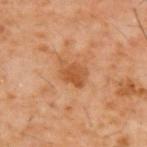biopsy status — catalogued during a skin exam; not biopsied
patient — male, aged around 60
lesion diameter — ~3.5 mm (longest diameter)
body site — the upper back
acquisition — ~15 mm tile from a whole-body skin photo